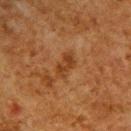workup: imaged on a skin check; not biopsied | TBP lesion metrics: a mean CIELAB color near L≈33 a*≈21 b*≈33, about 7 CIELAB-L* units darker than the surrounding skin, and a normalized border contrast of about 7; a classifier nevus-likeness of about 0/100 | tile lighting: cross-polarized | location: the upper back | patient: male, in their mid- to late 60s | imaging modality: total-body-photography crop, ~15 mm field of view.